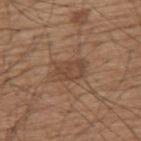Part of a total-body skin-imaging series; this lesion was reviewed on a skin check and was not flagged for biopsy.
The lesion is located on the upper back.
The tile uses white-light illumination.
The lesion's longest dimension is about 4 mm.
A lesion tile, about 15 mm wide, cut from a 3D total-body photograph.
A male subject, aged 73–77.
Automated image analysis of the tile measured a lesion area of about 8 mm², an eccentricity of roughly 0.8, and two-axis asymmetry of about 0.3.